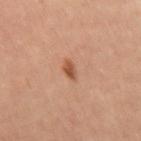Assessment: The lesion was photographed on a routine skin check and not biopsied; there is no pathology result. Acquisition and patient details: The total-body-photography lesion software estimated a footprint of about 2.5 mm² and a shape eccentricity near 0.8. The software also gave a detector confidence of about 100 out of 100 that the crop contains a lesion. The patient is a female aged approximately 40. A 15 mm crop from a total-body photograph taken for skin-cancer surveillance. Located on the right thigh.